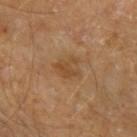Acquisition and patient details:
On the left forearm. The subject is a male in their 70s. A roughly 15 mm field-of-view crop from a total-body skin photograph.